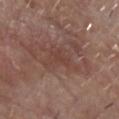Assessment: No biopsy was performed on this lesion — it was imaged during a full skin examination and was not determined to be concerning. Clinical summary: This is a white-light tile. A lesion tile, about 15 mm wide, cut from a 3D total-body photograph. An algorithmic analysis of the crop reported an average lesion color of about L≈42 a*≈19 b*≈24 (CIELAB) and a lesion–skin lightness drop of about 6. And it measured a border-irregularity index near 7/10, a color-variation rating of about 2/10, and peripheral color asymmetry of about 0.5. Located on the head or neck. The patient is a male aged around 80.